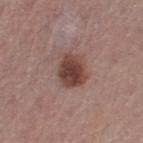Notes:
– workup — catalogued during a skin exam; not biopsied
– patient — female, aged approximately 55
– TBP lesion metrics — border irregularity of about 1.5 on a 0–10 scale, a color-variation rating of about 4/10, and radial color variation of about 1
– lesion diameter — ~4 mm (longest diameter)
– anatomic site — the left thigh
– image — ~15 mm tile from a whole-body skin photo